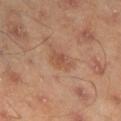  biopsy_status: not biopsied; imaged during a skin examination
  site: right lower leg
  patient:
    sex: male
    age_approx: 45
  image:
    source: total-body photography crop
    field_of_view_mm: 15